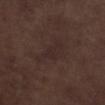No biopsy was performed on this lesion — it was imaged during a full skin examination and was not determined to be concerning.
Measured at roughly 5 mm in maximum diameter.
The lesion-visualizer software estimated a symmetry-axis asymmetry near 0.45. The software also gave a border-irregularity index near 5/10, a within-lesion color-variation index near 2/10, and peripheral color asymmetry of about 0.5.
This is a white-light tile.
On the left lower leg.
Cropped from a whole-body photographic skin survey; the tile spans about 15 mm.
A male subject, roughly 70 years of age.